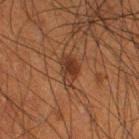Assessment:
No biopsy was performed on this lesion — it was imaged during a full skin examination and was not determined to be concerning.
Context:
Captured under cross-polarized illumination. Located on the leg. A close-up tile cropped from a whole-body skin photograph, about 15 mm across. The total-body-photography lesion software estimated a footprint of about 5.5 mm² and a symmetry-axis asymmetry near 0.35. The analysis additionally found border irregularity of about 3 on a 0–10 scale, internal color variation of about 3.5 on a 0–10 scale, and a peripheral color-asymmetry measure near 1. The subject is a male roughly 50 years of age. Longest diameter approximately 3.5 mm.